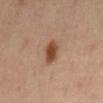Impression:
The lesion was tiled from a total-body skin photograph and was not biopsied.
Clinical summary:
A close-up tile cropped from a whole-body skin photograph, about 15 mm across. The total-body-photography lesion software estimated an area of roughly 6 mm² and a shape-asymmetry score of about 0.15 (0 = symmetric). The analysis additionally found a mean CIELAB color near L≈46 a*≈21 b*≈31. Located on the abdomen. A male subject aged around 55.